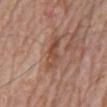biopsy status: no biopsy performed (imaged during a skin exam) | anatomic site: the mid back | lesion size: ≈5 mm | imaging modality: total-body-photography crop, ~15 mm field of view | tile lighting: white-light illumination | subject: male, about 80 years old.